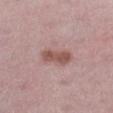Context:
About 4 mm across. Captured under white-light illumination. On the leg. The subject is a female about 45 years old. A roughly 15 mm field-of-view crop from a total-body skin photograph. The total-body-photography lesion software estimated a footprint of about 7 mm², a shape eccentricity near 0.9, and two-axis asymmetry of about 0.2. And it measured a mean CIELAB color near L≈53 a*≈21 b*≈22, about 11 CIELAB-L* units darker than the surrounding skin, and a lesion-to-skin contrast of about 8 (normalized; higher = more distinct). The software also gave an automated nevus-likeness rating near 50 out of 100 and lesion-presence confidence of about 100/100.